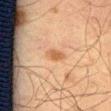<case>
<biopsy_status>not biopsied; imaged during a skin examination</biopsy_status>
<image>
  <source>total-body photography crop</source>
  <field_of_view_mm>15</field_of_view_mm>
</image>
<lighting>cross-polarized</lighting>
<site>left thigh</site>
<patient>
  <sex>male</sex>
  <age_approx>65</age_approx>
</patient>
</case>